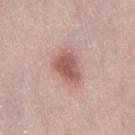No biopsy was performed on this lesion — it was imaged during a full skin examination and was not determined to be concerning.
Cropped from a whole-body photographic skin survey; the tile spans about 15 mm.
A female subject, aged 28–32.
Captured under white-light illumination.
The lesion's longest dimension is about 4 mm.
On the leg.
Automated image analysis of the tile measured a lesion area of about 9 mm², an eccentricity of roughly 0.8, and a shape-asymmetry score of about 0.25 (0 = symmetric). The software also gave a border-irregularity rating of about 2.5/10, a color-variation rating of about 4/10, and radial color variation of about 1. It also reported a nevus-likeness score of about 90/100.